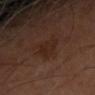Part of a total-body skin-imaging series; this lesion was reviewed on a skin check and was not flagged for biopsy.